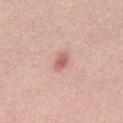Part of a total-body skin-imaging series; this lesion was reviewed on a skin check and was not flagged for biopsy. This image is a 15 mm lesion crop taken from a total-body photograph. This is a white-light tile. The total-body-photography lesion software estimated a mean CIELAB color near L≈61 a*≈26 b*≈23 and a lesion-to-skin contrast of about 7 (normalized; higher = more distinct). The analysis additionally found a nevus-likeness score of about 5/100 and a detector confidence of about 100 out of 100 that the crop contains a lesion. Approximately 3 mm at its widest. A male patient aged approximately 30.